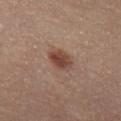Clinical impression:
The lesion was photographed on a routine skin check and not biopsied; there is no pathology result.
Clinical summary:
An algorithmic analysis of the crop reported roughly 11 lightness units darker than nearby skin. The software also gave a border-irregularity rating of about 2/10 and a peripheral color-asymmetry measure near 1.5. A female subject approximately 45 years of age. Cropped from a whole-body photographic skin survey; the tile spans about 15 mm. The tile uses cross-polarized illumination. Located on the left thigh. The lesion's longest dimension is about 3.5 mm.